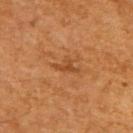Notes:
• follow-up · catalogued during a skin exam; not biopsied
• image-analysis metrics · a lesion color around L≈43 a*≈24 b*≈38 in CIELAB and a normalized border contrast of about 6; border irregularity of about 5.5 on a 0–10 scale, internal color variation of about 1 on a 0–10 scale, and a peripheral color-asymmetry measure near 0.5; a classifier nevus-likeness of about 0/100 and lesion-presence confidence of about 100/100
• size · about 3.5 mm
• image source · ~15 mm crop, total-body skin-cancer survey
• lighting · cross-polarized
• location · the back
• patient · male, aged approximately 60